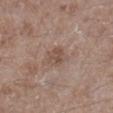Impression:
This lesion was catalogued during total-body skin photography and was not selected for biopsy.
Background:
A male patient, aged around 65. An algorithmic analysis of the crop reported a mean CIELAB color near L≈50 a*≈17 b*≈24 and a lesion-to-skin contrast of about 6 (normalized; higher = more distinct). And it measured a color-variation rating of about 3/10 and a peripheral color-asymmetry measure near 1. Captured under white-light illumination. Located on the right lower leg. A roughly 15 mm field-of-view crop from a total-body skin photograph. Longest diameter approximately 2.5 mm.